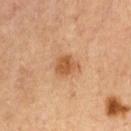Assessment:
The lesion was tiled from a total-body skin photograph and was not biopsied.
Clinical summary:
A male subject, in their mid- to late 60s. The total-body-photography lesion software estimated a footprint of about 5 mm², an eccentricity of roughly 0.45, and two-axis asymmetry of about 0.25. The analysis additionally found an average lesion color of about L≈50 a*≈22 b*≈36 (CIELAB), a lesion–skin lightness drop of about 10, and a normalized lesion–skin contrast near 8. The analysis additionally found a border-irregularity index near 2.5/10, a within-lesion color-variation index near 2/10, and a peripheral color-asymmetry measure near 0.5. A region of skin cropped from a whole-body photographic capture, roughly 15 mm wide. From the mid back. About 2.5 mm across.